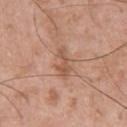The lesion was tiled from a total-body skin photograph and was not biopsied.